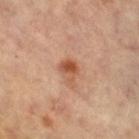The lesion was photographed on a routine skin check and not biopsied; there is no pathology result. A lesion tile, about 15 mm wide, cut from a 3D total-body photograph. A female subject, aged 63 to 67. The lesion is located on the leg. The lesion-visualizer software estimated an area of roughly 4.5 mm², an eccentricity of roughly 0.55, and a symmetry-axis asymmetry near 0.25. The analysis additionally found a lesion–skin lightness drop of about 10 and a normalized lesion–skin contrast near 8. It also reported a border-irregularity rating of about 2/10, a within-lesion color-variation index near 7.5/10, and peripheral color asymmetry of about 2.5. The software also gave a detector confidence of about 100 out of 100 that the crop contains a lesion. Measured at roughly 2.5 mm in maximum diameter. This is a cross-polarized tile.